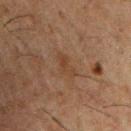Clinical impression: Imaged during a routine full-body skin examination; the lesion was not biopsied and no histopathology is available. Acquisition and patient details: From the chest. The patient is a male approximately 50 years of age. Captured under cross-polarized illumination. Cropped from a total-body skin-imaging series; the visible field is about 15 mm.